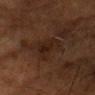notes = imaged on a skin check; not biopsied
illumination = cross-polarized illumination
anatomic site = the upper back
image source = ~15 mm tile from a whole-body skin photo
patient = male, aged around 55
lesion diameter = about 5 mm